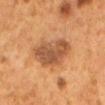Findings:
• notes: catalogued during a skin exam; not biopsied
• subject: female, aged approximately 50
• acquisition: ~15 mm crop, total-body skin-cancer survey
• anatomic site: the mid back